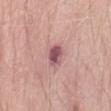Q: Was a biopsy performed?
A: no biopsy performed (imaged during a skin exam)
Q: Lesion size?
A: ≈2.5 mm
Q: How was this image acquired?
A: ~15 mm crop, total-body skin-cancer survey
Q: Automated lesion metrics?
A: peripheral color asymmetry of about 1
Q: What are the patient's age and sex?
A: male, roughly 80 years of age
Q: Where on the body is the lesion?
A: the front of the torso
Q: What lighting was used for the tile?
A: white-light